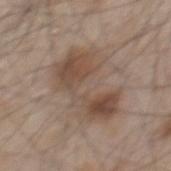{
  "patient": {
    "sex": "male",
    "age_approx": 45
  },
  "automated_metrics": {
    "area_mm2_approx": 24.0,
    "eccentricity": 0.85,
    "shape_asymmetry": 0.4,
    "cielab_L": 48,
    "cielab_a": 15,
    "cielab_b": 26,
    "vs_skin_darker_L": 9.0,
    "color_variation_0_10": 6.0,
    "peripheral_color_asymmetry": 2.0
  },
  "site": "mid back",
  "lighting": "white-light",
  "image": {
    "source": "total-body photography crop",
    "field_of_view_mm": 15
  }
}